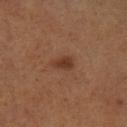Recorded during total-body skin imaging; not selected for excision or biopsy. The tile uses cross-polarized illumination. A male subject, roughly 55 years of age. Located on the left lower leg. A close-up tile cropped from a whole-body skin photograph, about 15 mm across.